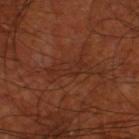Captured during whole-body skin photography for melanoma surveillance; the lesion was not biopsied. Automated image analysis of the tile measured a lesion color around L≈28 a*≈22 b*≈27 in CIELAB, roughly 4 lightness units darker than nearby skin, and a lesion-to-skin contrast of about 4.5 (normalized; higher = more distinct). The analysis additionally found border irregularity of about 6 on a 0–10 scale and a color-variation rating of about 2/10. It also reported a nevus-likeness score of about 0/100 and a lesion-detection confidence of about 80/100. Located on the left thigh. The recorded lesion diameter is about 4.5 mm. This image is a 15 mm lesion crop taken from a total-body photograph. A male patient, aged 68–72.